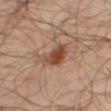workup = imaged on a skin check; not biopsied.